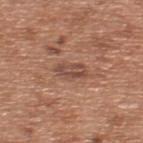Clinical impression: No biopsy was performed on this lesion — it was imaged during a full skin examination and was not determined to be concerning. Image and clinical context: The lesion is on the upper back. The patient is a male aged 63 to 67. Longest diameter approximately 3.5 mm. Cropped from a total-body skin-imaging series; the visible field is about 15 mm. The tile uses white-light illumination.